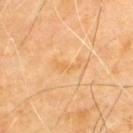{
  "biopsy_status": "not biopsied; imaged during a skin examination",
  "site": "upper back",
  "image": {
    "source": "total-body photography crop",
    "field_of_view_mm": 15
  },
  "patient": {
    "sex": "male",
    "age_approx": 70
  },
  "lesion_size": {
    "long_diameter_mm_approx": 3.0
  }
}